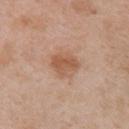Impression:
This lesion was catalogued during total-body skin photography and was not selected for biopsy.
Context:
Located on the arm. This image is a 15 mm lesion crop taken from a total-body photograph. The subject is a female aged around 40.